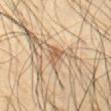| field | value |
|---|---|
| follow-up | no biopsy performed (imaged during a skin exam) |
| patient | male, about 65 years old |
| site | the front of the torso |
| acquisition | total-body-photography crop, ~15 mm field of view |
| tile lighting | cross-polarized |